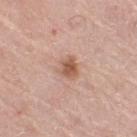Recorded during total-body skin imaging; not selected for excision or biopsy. The tile uses white-light illumination. A close-up tile cropped from a whole-body skin photograph, about 15 mm across. A male patient, aged around 80. An algorithmic analysis of the crop reported an automated nevus-likeness rating near 60 out of 100 and lesion-presence confidence of about 100/100. About 2.5 mm across. On the leg.